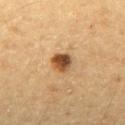  patient:
    sex: male
    age_approx: 60
  lighting: cross-polarized
  image:
    source: total-body photography crop
    field_of_view_mm: 15
  lesion_size:
    long_diameter_mm_approx: 2.5